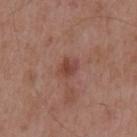{
  "lighting": "white-light",
  "image": {
    "source": "total-body photography crop",
    "field_of_view_mm": 15
  },
  "lesion_size": {
    "long_diameter_mm_approx": 2.5
  },
  "site": "mid back",
  "patient": {
    "sex": "male",
    "age_approx": 55
  }
}